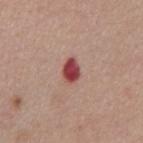Imaged during a routine full-body skin examination; the lesion was not biopsied and no histopathology is available. A male subject about 55 years old. A 15 mm close-up tile from a total-body photography series done for melanoma screening. This is a white-light tile. Longest diameter approximately 3 mm. The lesion is on the abdomen.